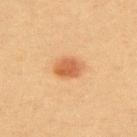The lesion was photographed on a routine skin check and not biopsied; there is no pathology result. A female subject, aged approximately 40. About 3.5 mm across. A 15 mm close-up tile from a total-body photography series done for melanoma screening. On the upper back. Imaged with cross-polarized lighting.